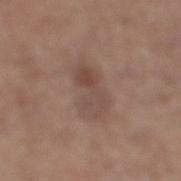<case>
  <biopsy_status>not biopsied; imaged during a skin examination</biopsy_status>
  <site>right lower leg</site>
  <lighting>white-light</lighting>
  <image>
    <source>total-body photography crop</source>
    <field_of_view_mm>15</field_of_view_mm>
  </image>
  <patient>
    <sex>male</sex>
    <age_approx>65</age_approx>
  </patient>
  <lesion_size>
    <long_diameter_mm_approx>6.0</long_diameter_mm_approx>
  </lesion_size>
  <automated_metrics>
    <eccentricity>0.85</eccentricity>
    <vs_skin_contrast_norm>5.0</vs_skin_contrast_norm>
    <nevus_likeness_0_100>5</nevus_likeness_0_100>
    <lesion_detection_confidence_0_100>100</lesion_detection_confidence_0_100>
  </automated_metrics>
</case>